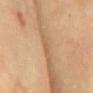Findings:
– workup · catalogued during a skin exam; not biopsied
– acquisition · ~15 mm crop, total-body skin-cancer survey
– diameter · ≈2 mm
– patient · male, approximately 70 years of age
– tile lighting · cross-polarized illumination
– site · the chest